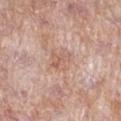A female subject, aged around 70. Automated tile analysis of the lesion measured a mean CIELAB color near L≈61 a*≈21 b*≈27, a lesion–skin lightness drop of about 7, and a normalized lesion–skin contrast near 5. And it measured a nevus-likeness score of about 0/100 and a detector confidence of about 100 out of 100 that the crop contains a lesion. A 15 mm crop from a total-body photograph taken for skin-cancer surveillance. On the leg. Imaged with white-light lighting. Approximately 3 mm at its widest.